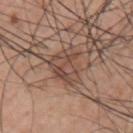Q: Was a biopsy performed?
A: imaged on a skin check; not biopsied